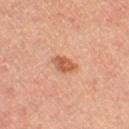biopsy status = no biopsy performed (imaged during a skin exam); acquisition = 15 mm crop, total-body photography; diameter = ≈2.5 mm; patient = female; location = the right thigh.